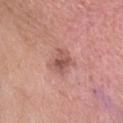Imaged during a routine full-body skin examination; the lesion was not biopsied and no histopathology is available.
This is a white-light tile.
Measured at roughly 3 mm in maximum diameter.
The lesion is located on the head or neck.
An algorithmic analysis of the crop reported a mean CIELAB color near L≈54 a*≈24 b*≈25, a lesion–skin lightness drop of about 10, and a normalized lesion–skin contrast near 7. And it measured a border-irregularity rating of about 2.5/10, a within-lesion color-variation index near 5/10, and radial color variation of about 2. The software also gave a nevus-likeness score of about 10/100 and a detector confidence of about 100 out of 100 that the crop contains a lesion.
Cropped from a total-body skin-imaging series; the visible field is about 15 mm.
A female patient roughly 55 years of age.